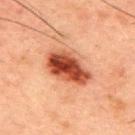lighting: cross-polarized illumination | imaging modality: ~15 mm tile from a whole-body skin photo | subject: male, aged around 50 | automated metrics: an average lesion color of about L≈39 a*≈26 b*≈30 (CIELAB), roughly 17 lightness units darker than nearby skin, and a lesion-to-skin contrast of about 13 (normalized; higher = more distinct); a border-irregularity index near 2.5/10, internal color variation of about 7 on a 0–10 scale, and a peripheral color-asymmetry measure near 2.5; a classifier nevus-likeness of about 90/100 and a detector confidence of about 100 out of 100 that the crop contains a lesion | lesion diameter: about 5.5 mm | location: the back.